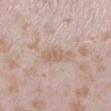workup: total-body-photography surveillance lesion; no biopsy
diameter: about 4 mm
site: the left lower leg
patient: female, aged 23–27
image: ~15 mm tile from a whole-body skin photo
image-analysis metrics: an area of roughly 5.5 mm², an eccentricity of roughly 0.9, and two-axis asymmetry of about 0.2